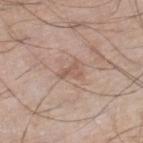Findings:
- follow-up — imaged on a skin check; not biopsied
- lesion diameter — ≈2.5 mm
- subject — male, in their 70s
- location — the leg
- image — ~15 mm crop, total-body skin-cancer survey
- tile lighting — white-light illumination
- TBP lesion metrics — a mean CIELAB color near L≈56 a*≈18 b*≈27, a lesion–skin lightness drop of about 8, and a lesion-to-skin contrast of about 5.5 (normalized; higher = more distinct); a classifier nevus-likeness of about 0/100